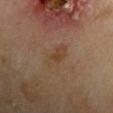{
  "biopsy_status": "not biopsied; imaged during a skin examination",
  "patient": {
    "sex": "male",
    "age_approx": 70
  },
  "lesion_size": {
    "long_diameter_mm_approx": 4.0
  },
  "lighting": "cross-polarized",
  "site": "left upper arm",
  "image": {
    "source": "total-body photography crop",
    "field_of_view_mm": 15
  }
}